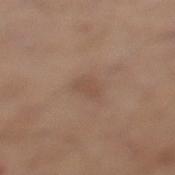The lesion was tiled from a total-body skin photograph and was not biopsied. Automated image analysis of the tile measured an area of roughly 3 mm², an outline eccentricity of about 0.9 (0 = round, 1 = elongated), and a symmetry-axis asymmetry near 0.3. And it measured a lesion–skin lightness drop of about 6 and a normalized lesion–skin contrast near 5. The analysis additionally found border irregularity of about 3 on a 0–10 scale and radial color variation of about 0. The analysis additionally found a nevus-likeness score of about 0/100 and a detector confidence of about 100 out of 100 that the crop contains a lesion. A male subject, in their mid- to late 60s. A 15 mm close-up tile from a total-body photography series done for melanoma screening. Located on the left lower leg. Longest diameter approximately 3 mm.